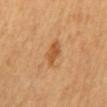biopsy_status: not biopsied; imaged during a skin examination
automated_metrics:
  cielab_L: 56
  cielab_a: 25
  cielab_b: 42
  border_irregularity_0_10: 2.5
  color_variation_0_10: 2.5
  peripheral_color_asymmetry: 1.0
image:
  source: total-body photography crop
  field_of_view_mm: 15
site: back
patient:
  sex: female
  age_approx: 60
lesion_size:
  long_diameter_mm_approx: 3.5
lighting: cross-polarized